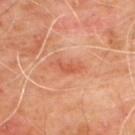<lesion>
<patient>
  <sex>male</sex>
  <age_approx>65</age_approx>
</patient>
<site>upper back</site>
<image>
  <source>total-body photography crop</source>
  <field_of_view_mm>15</field_of_view_mm>
</image>
<lighting>cross-polarized</lighting>
<lesion_size>
  <long_diameter_mm_approx>2.5</long_diameter_mm_approx>
</lesion_size>
</lesion>